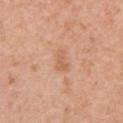Q: Is there a histopathology result?
A: no biopsy performed (imaged during a skin exam)
Q: What is the anatomic site?
A: the right upper arm
Q: Patient demographics?
A: female, roughly 60 years of age
Q: What is the imaging modality?
A: total-body-photography crop, ~15 mm field of view